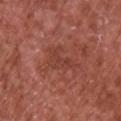{"biopsy_status": "not biopsied; imaged during a skin examination", "automated_metrics": {"shape_asymmetry": 0.6, "vs_skin_darker_L": 6.0, "vs_skin_contrast_norm": 4.5, "nevus_likeness_0_100": 0, "lesion_detection_confidence_0_100": 100}, "image": {"source": "total-body photography crop", "field_of_view_mm": 15}, "lesion_size": {"long_diameter_mm_approx": 3.5}, "patient": {"sex": "male", "age_approx": 65}, "site": "chest", "lighting": "white-light"}